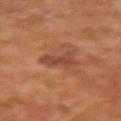<lesion>
<biopsy_status>not biopsied; imaged during a skin examination</biopsy_status>
<patient>
  <sex>male</sex>
  <age_approx>65</age_approx>
</patient>
<lighting>cross-polarized</lighting>
<site>right upper arm</site>
<image>
  <source>total-body photography crop</source>
  <field_of_view_mm>15</field_of_view_mm>
</image>
<lesion_size>
  <long_diameter_mm_approx>3.5</long_diameter_mm_approx>
</lesion_size>
</lesion>